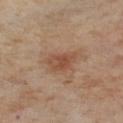Case summary:
- image source: 15 mm crop, total-body photography
- image-analysis metrics: a footprint of about 6.5 mm², an outline eccentricity of about 0.75 (0 = round, 1 = elongated), and two-axis asymmetry of about 0.3; border irregularity of about 3 on a 0–10 scale, a color-variation rating of about 3/10, and radial color variation of about 1
- patient: female, aged around 55
- anatomic site: the left lower leg
- diameter: ≈3.5 mm